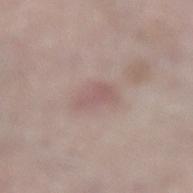notes=total-body-photography surveillance lesion; no biopsy
lighting=white-light
image source=~15 mm tile from a whole-body skin photo
body site=the left lower leg
patient=male, about 65 years old